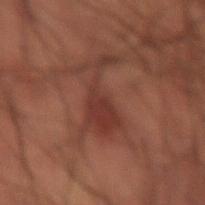Located on the lower back. The patient is a male roughly 50 years of age. A lesion tile, about 15 mm wide, cut from a 3D total-body photograph.